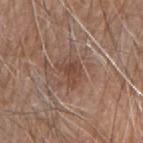Q: Is there a histopathology result?
A: total-body-photography surveillance lesion; no biopsy
Q: What kind of image is this?
A: ~15 mm tile from a whole-body skin photo
Q: How was the tile lit?
A: white-light illumination
Q: Patient demographics?
A: male, in their 80s
Q: What did automated image analysis measure?
A: a shape eccentricity near 0.6; about 8 CIELAB-L* units darker than the surrounding skin and a normalized lesion–skin contrast near 6.5; internal color variation of about 2.5 on a 0–10 scale and radial color variation of about 1
Q: Lesion location?
A: the right forearm
Q: Lesion size?
A: ~3 mm (longest diameter)